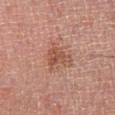body site = the leg
subject = male, aged around 65
acquisition = ~15 mm crop, total-body skin-cancer survey
diameter = ~3.5 mm (longest diameter)
illumination = cross-polarized illumination